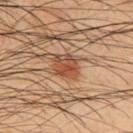Impression:
The lesion was photographed on a routine skin check and not biopsied; there is no pathology result.
Clinical summary:
On the upper back. The total-body-photography lesion software estimated a lesion area of about 8 mm². The analysis additionally found a lesion color around L≈47 a*≈22 b*≈32 in CIELAB, about 10 CIELAB-L* units darker than the surrounding skin, and a lesion-to-skin contrast of about 8 (normalized; higher = more distinct). A lesion tile, about 15 mm wide, cut from a 3D total-body photograph. About 3 mm across. A male patient, about 50 years old.